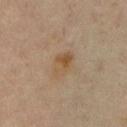Assessment: The lesion was photographed on a routine skin check and not biopsied; there is no pathology result. Clinical summary: Located on the front of the torso. A lesion tile, about 15 mm wide, cut from a 3D total-body photograph. Captured under cross-polarized illumination. A female subject, about 45 years old.